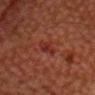Captured under cross-polarized illumination. On the head or neck. About 3.5 mm across. A male subject, aged 63 to 67. Cropped from a total-body skin-imaging series; the visible field is about 15 mm.